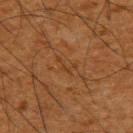| field | value |
|---|---|
| notes | total-body-photography surveillance lesion; no biopsy |
| patient | male, about 65 years old |
| image source | ~15 mm crop, total-body skin-cancer survey |
| site | the upper back |